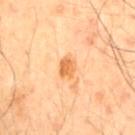Q: Was a biopsy performed?
A: total-body-photography surveillance lesion; no biopsy
Q: Who is the patient?
A: male, in their 50s
Q: How was this image acquired?
A: 15 mm crop, total-body photography
Q: Lesion location?
A: the chest